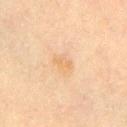No biopsy was performed on this lesion — it was imaged during a full skin examination and was not determined to be concerning.
The total-body-photography lesion software estimated a footprint of about 3 mm², a shape eccentricity near 0.85, and a symmetry-axis asymmetry near 0.3. It also reported a lesion–skin lightness drop of about 5 and a lesion-to-skin contrast of about 5 (normalized; higher = more distinct). It also reported a border-irregularity index near 3/10 and a within-lesion color-variation index near 2/10.
A female patient, roughly 60 years of age.
The lesion is located on the chest.
A 15 mm close-up tile from a total-body photography series done for melanoma screening.
Measured at roughly 2.5 mm in maximum diameter.
Captured under cross-polarized illumination.